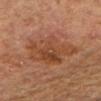<tbp_lesion>
  <image>
    <source>total-body photography crop</source>
    <field_of_view_mm>15</field_of_view_mm>
  </image>
  <patient>
    <sex>male</sex>
    <age_approx>65</age_approx>
  </patient>
  <automated_metrics>
    <cielab_L>40</cielab_L>
    <cielab_a>21</cielab_a>
    <cielab_b>30</cielab_b>
    <vs_skin_darker_L>7.0</vs_skin_darker_L>
    <nevus_likeness_0_100>0</nevus_likeness_0_100>
    <lesion_detection_confidence_0_100>100</lesion_detection_confidence_0_100>
  </automated_metrics>
  <lesion_size>
    <long_diameter_mm_approx>6.5</long_diameter_mm_approx>
  </lesion_size>
  <site>right forearm</site>
</tbp_lesion>